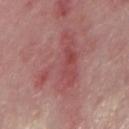<tbp_lesion>
<biopsy_status>not biopsied; imaged during a skin examination</biopsy_status>
<image>
  <source>total-body photography crop</source>
  <field_of_view_mm>15</field_of_view_mm>
</image>
<site>right forearm</site>
<patient>
  <sex>male</sex>
  <age_approx>75</age_approx>
</patient>
</tbp_lesion>